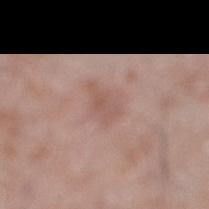<lesion>
<biopsy_status>not biopsied; imaged during a skin examination</biopsy_status>
<site>leg</site>
<image>
  <source>total-body photography crop</source>
  <field_of_view_mm>15</field_of_view_mm>
</image>
<patient>
  <sex>male</sex>
  <age_approx>60</age_approx>
</patient>
</lesion>